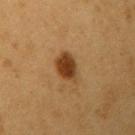No biopsy was performed on this lesion — it was imaged during a full skin examination and was not determined to be concerning. Captured under cross-polarized illumination. The lesion's longest dimension is about 3.5 mm. A male subject, aged approximately 55. From the right upper arm. A region of skin cropped from a whole-body photographic capture, roughly 15 mm wide.